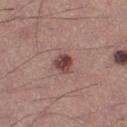Part of a total-body skin-imaging series; this lesion was reviewed on a skin check and was not flagged for biopsy.
A 15 mm close-up extracted from a 3D total-body photography capture.
Approximately 2.5 mm at its widest.
A male subject, aged 38–42.
From the left thigh.